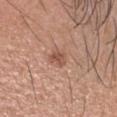Part of a total-body skin-imaging series; this lesion was reviewed on a skin check and was not flagged for biopsy. Cropped from a total-body skin-imaging series; the visible field is about 15 mm. The lesion is located on the head or neck. A male subject aged 38–42.